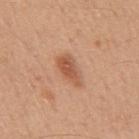Impression: Captured during whole-body skin photography for melanoma surveillance; the lesion was not biopsied. Clinical summary: The tile uses white-light illumination. The subject is a male aged 48–52. A roughly 15 mm field-of-view crop from a total-body skin photograph. Automated tile analysis of the lesion measured a lesion area of about 6.5 mm², an eccentricity of roughly 0.85, and a symmetry-axis asymmetry near 0.25. The analysis additionally found a border-irregularity rating of about 2.5/10, internal color variation of about 3 on a 0–10 scale, and peripheral color asymmetry of about 1. Approximately 4 mm at its widest. Located on the mid back.